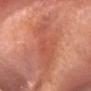Q: Was this lesion biopsied?
A: no biopsy performed (imaged during a skin exam)
Q: Lesion location?
A: the head or neck
Q: Who is the patient?
A: female, in their mid-50s
Q: What did automated image analysis measure?
A: a lesion area of about 21 mm², a shape eccentricity near 0.9, and a shape-asymmetry score of about 0.35 (0 = symmetric); a nevus-likeness score of about 0/100 and lesion-presence confidence of about 75/100
Q: What is the imaging modality?
A: ~15 mm crop, total-body skin-cancer survey
Q: Illumination type?
A: white-light illumination